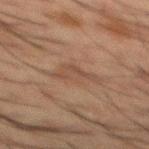• notes — total-body-photography surveillance lesion; no biopsy
• lesion diameter — about 4.5 mm
• image source — ~15 mm tile from a whole-body skin photo
• anatomic site — the mid back
• tile lighting — cross-polarized illumination
• subject — male, in their 50s